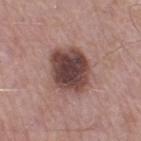Impression: Captured during whole-body skin photography for melanoma surveillance; the lesion was not biopsied. Background: The subject is a male aged 53 to 57. A close-up tile cropped from a whole-body skin photograph, about 15 mm across. Automated tile analysis of the lesion measured a shape eccentricity near 0.55 and a shape-asymmetry score of about 0.1 (0 = symmetric). The software also gave border irregularity of about 1.5 on a 0–10 scale, a color-variation rating of about 5.5/10, and peripheral color asymmetry of about 1.5. Approximately 5.5 mm at its widest. The lesion is on the right thigh. Captured under white-light illumination.